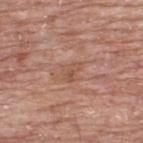<record>
  <biopsy_status>not biopsied; imaged during a skin examination</biopsy_status>
  <automated_metrics>
    <area_mm2_approx>3.5</area_mm2_approx>
    <eccentricity>0.75</eccentricity>
    <shape_asymmetry>0.35</shape_asymmetry>
    <cielab_L>53</cielab_L>
    <cielab_a>21</cielab_a>
    <cielab_b>29</cielab_b>
    <vs_skin_darker_L>7.0</vs_skin_darker_L>
    <vs_skin_contrast_norm>5.5</vs_skin_contrast_norm>
  </automated_metrics>
  <lesion_size>
    <long_diameter_mm_approx>3.0</long_diameter_mm_approx>
  </lesion_size>
  <lighting>white-light</lighting>
  <patient>
    <sex>male</sex>
    <age_approx>65</age_approx>
  </patient>
  <site>upper back</site>
  <image>
    <source>total-body photography crop</source>
    <field_of_view_mm>15</field_of_view_mm>
  </image>
</record>